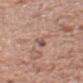Impression:
The lesion was tiled from a total-body skin photograph and was not biopsied.
Background:
A close-up tile cropped from a whole-body skin photograph, about 15 mm across. From the chest. A male patient, aged approximately 70.